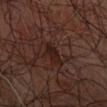Assessment:
The lesion was tiled from a total-body skin photograph and was not biopsied.
Acquisition and patient details:
A lesion tile, about 15 mm wide, cut from a 3D total-body photograph. About 3 mm across. The lesion-visualizer software estimated roughly 6 lightness units darker than nearby skin. The software also gave border irregularity of about 2.5 on a 0–10 scale. Imaged with cross-polarized lighting. A male patient, aged around 65. From the left forearm.